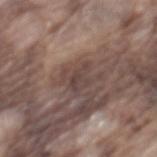Impression: Part of a total-body skin-imaging series; this lesion was reviewed on a skin check and was not flagged for biopsy.